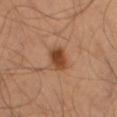notes: no biopsy performed (imaged during a skin exam) | lighting: cross-polarized | body site: the left thigh | subject: male, roughly 50 years of age | image-analysis metrics: a footprint of about 5.5 mm², an outline eccentricity of about 0.75 (0 = round, 1 = elongated), and two-axis asymmetry of about 0.2; an average lesion color of about L≈43 a*≈23 b*≈34 (CIELAB), a lesion–skin lightness drop of about 13, and a normalized lesion–skin contrast near 10; a nevus-likeness score of about 100/100 and a lesion-detection confidence of about 100/100 | lesion size: ~3 mm (longest diameter) | imaging modality: 15 mm crop, total-body photography.